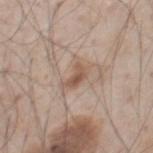Case summary:
- notes · catalogued during a skin exam; not biopsied
- anatomic site · the chest
- patient · male, approximately 60 years of age
- TBP lesion metrics · an outline eccentricity of about 0.85 (0 = round, 1 = elongated) and two-axis asymmetry of about 0.4; a color-variation rating of about 3/10; an automated nevus-likeness rating near 10 out of 100
- image source · ~15 mm crop, total-body skin-cancer survey
- illumination · white-light illumination